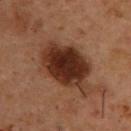<record>
<biopsy_status>not biopsied; imaged during a skin examination</biopsy_status>
<image>
  <source>total-body photography crop</source>
  <field_of_view_mm>15</field_of_view_mm>
</image>
<patient>
  <sex>male</sex>
  <age_approx>55</age_approx>
</patient>
<site>upper back</site>
<lighting>cross-polarized</lighting>
<lesion_size>
  <long_diameter_mm_approx>8.0</long_diameter_mm_approx>
</lesion_size>
</record>